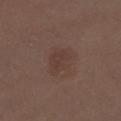<lesion>
<biopsy_status>not biopsied; imaged during a skin examination</biopsy_status>
<automated_metrics>
  <cielab_L>37</cielab_L>
  <cielab_a>16</cielab_a>
  <cielab_b>21</cielab_b>
  <vs_skin_darker_L>5.0</vs_skin_darker_L>
  <border_irregularity_0_10>2.5</border_irregularity_0_10>
  <color_variation_0_10>1.5</color_variation_0_10>
  <peripheral_color_asymmetry>0.5</peripheral_color_asymmetry>
  <nevus_likeness_0_100>10</nevus_likeness_0_100>
</automated_metrics>
<site>right lower leg</site>
<lighting>white-light</lighting>
<image>
  <source>total-body photography crop</source>
  <field_of_view_mm>15</field_of_view_mm>
</image>
<lesion_size>
  <long_diameter_mm_approx>3.5</long_diameter_mm_approx>
</lesion_size>
<patient>
  <sex>female</sex>
  <age_approx>40</age_approx>
</patient>
</lesion>